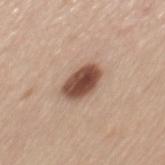Impression: No biopsy was performed on this lesion — it was imaged during a full skin examination and was not determined to be concerning. Clinical summary: Measured at roughly 4 mm in maximum diameter. A female patient roughly 55 years of age. Cropped from a whole-body photographic skin survey; the tile spans about 15 mm. The lesion-visualizer software estimated an average lesion color of about L≈48 a*≈20 b*≈27 (CIELAB), roughly 19 lightness units darker than nearby skin, and a lesion-to-skin contrast of about 13 (normalized; higher = more distinct). On the mid back. Captured under white-light illumination.